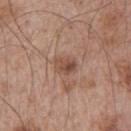The lesion was photographed on a routine skin check and not biopsied; there is no pathology result.
A region of skin cropped from a whole-body photographic capture, roughly 15 mm wide.
Located on the left upper arm.
The patient is a male in their mid- to late 60s.